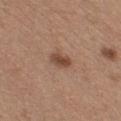biopsy status = catalogued during a skin exam; not biopsied | subject = female, in their 30s | imaging modality = ~15 mm crop, total-body skin-cancer survey | lesion size = ~2.5 mm (longest diameter) | location = the chest.